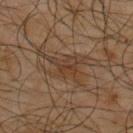follow-up = total-body-photography surveillance lesion; no biopsy
subject = male, in their mid- to late 60s
image = 15 mm crop, total-body photography
anatomic site = the upper back
lesion size = about 6 mm